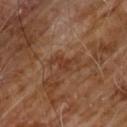| field | value |
|---|---|
| follow-up | no biopsy performed (imaged during a skin exam) |
| lighting | cross-polarized illumination |
| imaging modality | total-body-photography crop, ~15 mm field of view |
| subject | male, aged 58 to 62 |
| location | the front of the torso |